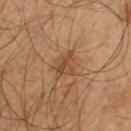Captured under cross-polarized illumination.
This image is a 15 mm lesion crop taken from a total-body photograph.
The lesion is located on the left upper arm.
A male subject, roughly 65 years of age.